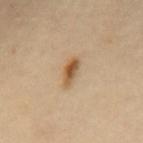The lesion is located on the mid back. A female patient aged 58–62. A 15 mm crop from a total-body photograph taken for skin-cancer surveillance. Imaged with cross-polarized lighting.